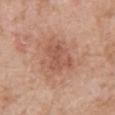<case>
  <biopsy_status>not biopsied; imaged during a skin examination</biopsy_status>
  <patient>
    <sex>male</sex>
    <age_approx>60</age_approx>
  </patient>
  <site>back</site>
  <image>
    <source>total-body photography crop</source>
    <field_of_view_mm>15</field_of_view_mm>
  </image>
  <lesion_size>
    <long_diameter_mm_approx>3.5</long_diameter_mm_approx>
  </lesion_size>
  <automated_metrics>
    <cielab_L>54</cielab_L>
    <cielab_a>24</cielab_a>
    <cielab_b>30</cielab_b>
    <vs_skin_darker_L>7.0</vs_skin_darker_L>
    <vs_skin_contrast_norm>5.0</vs_skin_contrast_norm>
    <nevus_likeness_0_100>0</nevus_likeness_0_100>
    <lesion_detection_confidence_0_100>100</lesion_detection_confidence_0_100>
  </automated_metrics>
</case>